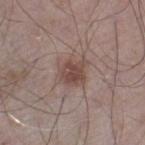Notes:
- workup — total-body-photography surveillance lesion; no biopsy
- patient — male, in their mid-60s
- anatomic site — the left thigh
- image source — ~15 mm tile from a whole-body skin photo
- lesion size — ≈4 mm